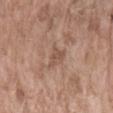  biopsy_status: not biopsied; imaged during a skin examination
  image:
    source: total-body photography crop
    field_of_view_mm: 15
  site: arm
  automated_metrics:
    cielab_L: 51
    cielab_a: 19
    cielab_b: 26
    vs_skin_darker_L: 8.0
    vs_skin_contrast_norm: 5.5
    border_irregularity_0_10: 4.5
    color_variation_0_10: 0.0
    peripheral_color_asymmetry: 0.0
    nevus_likeness_0_100: 0
    lesion_detection_confidence_0_100: 100
  lesion_size:
    long_diameter_mm_approx: 2.5
  lighting: white-light
  patient:
    sex: female
    age_approx: 75